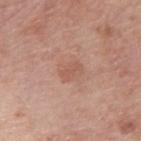Impression:
Recorded during total-body skin imaging; not selected for excision or biopsy.
Context:
The lesion is on the left upper arm. A close-up tile cropped from a whole-body skin photograph, about 15 mm across. Imaged with white-light lighting. A male patient in their mid-50s. The total-body-photography lesion software estimated a lesion area of about 5.5 mm², an outline eccentricity of about 0.55 (0 = round, 1 = elongated), and two-axis asymmetry of about 0.2. The analysis additionally found a lesion color around L≈56 a*≈22 b*≈28 in CIELAB and a lesion–skin lightness drop of about 6. The software also gave an automated nevus-likeness rating near 5 out of 100 and a detector confidence of about 100 out of 100 that the crop contains a lesion. Measured at roughly 3 mm in maximum diameter.